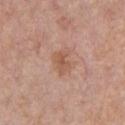This lesion was catalogued during total-body skin photography and was not selected for biopsy. Automated image analysis of the tile measured an area of roughly 4.5 mm² and two-axis asymmetry of about 0.35. It also reported a border-irregularity rating of about 3.5/10, a color-variation rating of about 2/10, and a peripheral color-asymmetry measure near 0.5. It also reported a nevus-likeness score of about 0/100 and a detector confidence of about 100 out of 100 that the crop contains a lesion. The lesion's longest dimension is about 3 mm. A male subject in their 60s. Cropped from a total-body skin-imaging series; the visible field is about 15 mm. This is a white-light tile. On the chest.